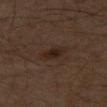Recorded during total-body skin imaging; not selected for excision or biopsy.